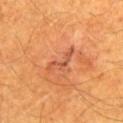biopsy_status: not biopsied; imaged during a skin examination
site: front of the torso
image:
  source: total-body photography crop
  field_of_view_mm: 15
patient:
  sex: male
  age_approx: 60
lighting: cross-polarized
automated_metrics:
  cielab_L: 50
  cielab_a: 28
  cielab_b: 36
  vs_skin_darker_L: 8.0
  vs_skin_contrast_norm: 6.0
  border_irregularity_0_10: 7.5
  color_variation_0_10: 0.0
  peripheral_color_asymmetry: 0.0
lesion_size:
  long_diameter_mm_approx: 4.0